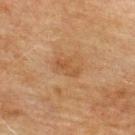Acquisition and patient details:
A lesion tile, about 15 mm wide, cut from a 3D total-body photograph. The lesion-visualizer software estimated a lesion area of about 4.5 mm². It also reported a mean CIELAB color near L≈43 a*≈18 b*≈32, roughly 6 lightness units darker than nearby skin, and a normalized border contrast of about 5. It also reported a classifier nevus-likeness of about 0/100 and a lesion-detection confidence of about 100/100. Captured under cross-polarized illumination. The lesion's longest dimension is about 3 mm. Located on the upper back. The patient is a male in their mid- to late 70s.